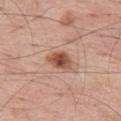biopsy status = total-body-photography surveillance lesion; no biopsy | subject = male, roughly 55 years of age | anatomic site = the left thigh | image source = ~15 mm tile from a whole-body skin photo.